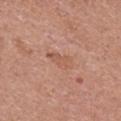| key | value |
|---|---|
| notes | imaged on a skin check; not biopsied |
| image | ~15 mm tile from a whole-body skin photo |
| body site | the chest |
| patient | female, aged 48 to 52 |
| illumination | white-light |